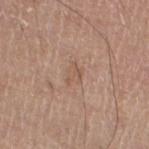<tbp_lesion>
<biopsy_status>not biopsied; imaged during a skin examination</biopsy_status>
<lesion_size>
  <long_diameter_mm_approx>2.5</long_diameter_mm_approx>
</lesion_size>
<automated_metrics>
  <border_irregularity_0_10>4.5</border_irregularity_0_10>
  <color_variation_0_10>1.5</color_variation_0_10>
  <peripheral_color_asymmetry>0.5</peripheral_color_asymmetry>
</automated_metrics>
<patient>
  <sex>male</sex>
  <age_approx>65</age_approx>
</patient>
<image>
  <source>total-body photography crop</source>
  <field_of_view_mm>15</field_of_view_mm>
</image>
<site>left lower leg</site>
<lighting>white-light</lighting>
</tbp_lesion>